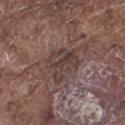workup = catalogued during a skin exam; not biopsied